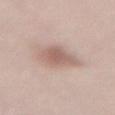Findings:
– workup — no biopsy performed (imaged during a skin exam)
– diameter — ~4.5 mm (longest diameter)
– anatomic site — the back
– imaging modality — ~15 mm tile from a whole-body skin photo
– subject — female, roughly 65 years of age
– automated lesion analysis — a border-irregularity index near 2.5/10, a color-variation rating of about 3/10, and peripheral color asymmetry of about 1; a classifier nevus-likeness of about 45/100